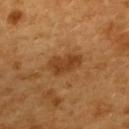biopsy_status: not biopsied; imaged during a skin examination
site: upper back
patient:
  sex: female
  age_approx: 55
image:
  source: total-body photography crop
  field_of_view_mm: 15
lesion_size:
  long_diameter_mm_approx: 4.0